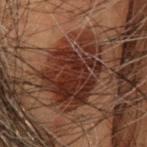Captured during whole-body skin photography for melanoma surveillance; the lesion was not biopsied. Automated tile analysis of the lesion measured a mean CIELAB color near L≈24 a*≈19 b*≈22 and roughly 11 lightness units darker than nearby skin. And it measured a nevus-likeness score of about 55/100 and a detector confidence of about 100 out of 100 that the crop contains a lesion. Cropped from a total-body skin-imaging series; the visible field is about 15 mm. Imaged with cross-polarized lighting. Located on the head or neck. A male patient approximately 55 years of age.